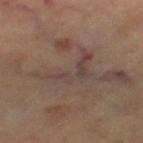Assessment: This lesion was catalogued during total-body skin photography and was not selected for biopsy. Acquisition and patient details: On the left thigh. The subject is aged 58 to 62. A lesion tile, about 15 mm wide, cut from a 3D total-body photograph. Longest diameter approximately 6.5 mm. Captured under cross-polarized illumination.